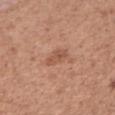{
  "image": {
    "source": "total-body photography crop",
    "field_of_view_mm": 15
  },
  "patient": {
    "sex": "female",
    "age_approx": 55
  },
  "site": "right upper arm"
}